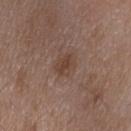| key | value |
|---|---|
| biopsy status | no biopsy performed (imaged during a skin exam) |
| acquisition | 15 mm crop, total-body photography |
| illumination | white-light illumination |
| patient | female, aged 68 to 72 |
| lesion diameter | about 3 mm |
| location | the upper back |